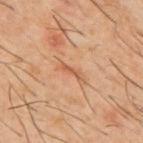<record>
<biopsy_status>not biopsied; imaged during a skin examination</biopsy_status>
<image>
  <source>total-body photography crop</source>
  <field_of_view_mm>15</field_of_view_mm>
</image>
<site>upper back</site>
<patient>
  <sex>male</sex>
  <age_approx>60</age_approx>
</patient>
</record>